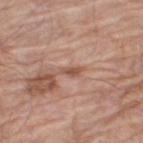Part of a total-body skin-imaging series; this lesion was reviewed on a skin check and was not flagged for biopsy.